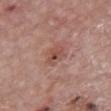Q: Is there a histopathology result?
A: no biopsy performed (imaged during a skin exam)
Q: Lesion location?
A: the chest
Q: Automated lesion metrics?
A: a shape eccentricity near 0.75 and a shape-asymmetry score of about 0.25 (0 = symmetric); a border-irregularity rating of about 3/10, a color-variation rating of about 3.5/10, and radial color variation of about 1.5
Q: What kind of image is this?
A: ~15 mm crop, total-body skin-cancer survey
Q: What lighting was used for the tile?
A: white-light illumination
Q: What are the patient's age and sex?
A: male, approximately 85 years of age or older